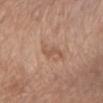Impression:
Part of a total-body skin-imaging series; this lesion was reviewed on a skin check and was not flagged for biopsy.
Context:
The tile uses white-light illumination. Cropped from a whole-body photographic skin survey; the tile spans about 15 mm. Measured at roughly 2.5 mm in maximum diameter. A male patient, aged 68 to 72. On the front of the torso. Automated tile analysis of the lesion measured a lesion area of about 2.5 mm². The software also gave a border-irregularity index near 4.5/10, a color-variation rating of about 0/10, and a peripheral color-asymmetry measure near 0. The analysis additionally found lesion-presence confidence of about 100/100.